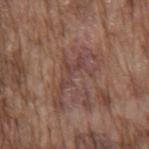Clinical impression:
No biopsy was performed on this lesion — it was imaged during a full skin examination and was not determined to be concerning.
Image and clinical context:
This image is a 15 mm lesion crop taken from a total-body photograph. Longest diameter approximately 7 mm. The lesion is located on the mid back. A male subject aged 73 to 77.